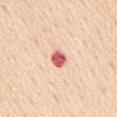Clinical impression: The lesion was tiled from a total-body skin photograph and was not biopsied. Image and clinical context: The subject is a male about 60 years old. Longest diameter approximately 2.5 mm. An algorithmic analysis of the crop reported a lesion color around L≈64 a*≈34 b*≈28 in CIELAB, a lesion–skin lightness drop of about 20, and a lesion-to-skin contrast of about 11.5 (normalized; higher = more distinct). The software also gave a border-irregularity rating of about 1/10, a color-variation rating of about 3/10, and radial color variation of about 1. The analysis additionally found a classifier nevus-likeness of about 0/100 and a lesion-detection confidence of about 100/100. Imaged with white-light lighting. From the mid back. Cropped from a total-body skin-imaging series; the visible field is about 15 mm.